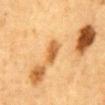Q: How large is the lesion?
A: ≈3 mm
Q: What did automated image analysis measure?
A: an area of roughly 5 mm²; a border-irregularity rating of about 2.5/10, a color-variation rating of about 3.5/10, and a peripheral color-asymmetry measure near 1; a nevus-likeness score of about 90/100 and a detector confidence of about 100 out of 100 that the crop contains a lesion
Q: How was this image acquired?
A: ~15 mm tile from a whole-body skin photo
Q: How was the tile lit?
A: cross-polarized illumination
Q: What are the patient's age and sex?
A: male, approximately 85 years of age or older
Q: Where on the body is the lesion?
A: the abdomen